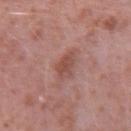Part of a total-body skin-imaging series; this lesion was reviewed on a skin check and was not flagged for biopsy. From the arm. About 3 mm across. Cropped from a total-body skin-imaging series; the visible field is about 15 mm. A male patient roughly 40 years of age.